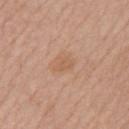Background: This image is a 15 mm lesion crop taken from a total-body photograph. Longest diameter approximately 2.5 mm. An algorithmic analysis of the crop reported a border-irregularity rating of about 2.5/10, a color-variation rating of about 1.5/10, and a peripheral color-asymmetry measure near 0.5. On the arm. The patient is a female in their mid- to late 60s.